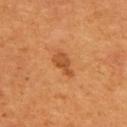| field | value |
|---|---|
| biopsy status | no biopsy performed (imaged during a skin exam) |
| diameter | ≈3.5 mm |
| patient | male, about 55 years old |
| location | the upper back |
| image source | 15 mm crop, total-body photography |
| illumination | cross-polarized |